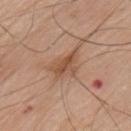Findings:
- workup · no biopsy performed (imaged during a skin exam)
- patient · male, about 80 years old
- acquisition · ~15 mm crop, total-body skin-cancer survey
- illumination · white-light
- lesion diameter · ≈4 mm
- image-analysis metrics · a mean CIELAB color near L≈52 a*≈20 b*≈31, a lesion–skin lightness drop of about 9, and a normalized border contrast of about 7
- location · the chest